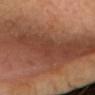image-analysis metrics: a mean CIELAB color near L≈40 a*≈24 b*≈30 and a normalized border contrast of about 3; border irregularity of about 3 on a 0–10 scale, a color-variation rating of about 0/10, and a peripheral color-asymmetry measure near 0; an automated nevus-likeness rating near 0 out of 100 and a lesion-detection confidence of about 95/100 | site: the head or neck | acquisition: ~15 mm crop, total-body skin-cancer survey | illumination: cross-polarized illumination | subject: female, aged approximately 70.